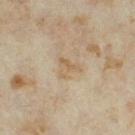This lesion was catalogued during total-body skin photography and was not selected for biopsy. On the right thigh. The lesion's longest dimension is about 3.5 mm. A 15 mm close-up extracted from a 3D total-body photography capture. A female patient in their mid-30s.